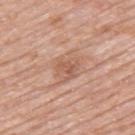Clinical summary:
From the upper back. The tile uses white-light illumination. Cropped from a total-body skin-imaging series; the visible field is about 15 mm. The lesion's longest dimension is about 4 mm. The lesion-visualizer software estimated a footprint of about 9 mm² and a symmetry-axis asymmetry near 0.25. The analysis additionally found internal color variation of about 3.5 on a 0–10 scale and peripheral color asymmetry of about 1. The patient is a male approximately 80 years of age.